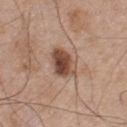Image and clinical context:
A male patient, roughly 55 years of age. Measured at roughly 3.5 mm in maximum diameter. This image is a 15 mm lesion crop taken from a total-body photograph. Located on the upper back.